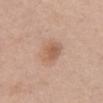notes: imaged on a skin check; not biopsied
subject: female, about 50 years old
lesion diameter: about 3.5 mm
location: the abdomen
imaging modality: ~15 mm crop, total-body skin-cancer survey
tile lighting: white-light illumination